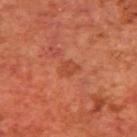Findings:
* workup — catalogued during a skin exam; not biopsied
* TBP lesion metrics — an area of roughly 4 mm², an outline eccentricity of about 0.65 (0 = round, 1 = elongated), and a symmetry-axis asymmetry near 0.3; roughly 6 lightness units darker than nearby skin and a normalized lesion–skin contrast near 5; internal color variation of about 1.5 on a 0–10 scale and a peripheral color-asymmetry measure near 0.5; a classifier nevus-likeness of about 5/100 and lesion-presence confidence of about 100/100
* size — ~2.5 mm (longest diameter)
* body site — the upper back
* lighting — cross-polarized
* imaging modality — 15 mm crop, total-body photography
* patient — male, aged approximately 70